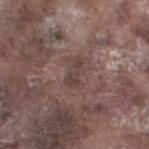workup: total-body-photography surveillance lesion; no biopsy | subject: male, aged approximately 75 | image: ~15 mm crop, total-body skin-cancer survey | location: the left thigh | lighting: white-light | lesion size: ~3 mm (longest diameter).